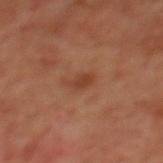Assessment:
This lesion was catalogued during total-body skin photography and was not selected for biopsy.
Context:
Cropped from a total-body skin-imaging series; the visible field is about 15 mm. A male patient about 70 years old. Located on the mid back.